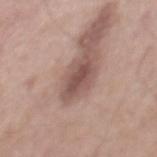<lesion>
<biopsy_status>not biopsied; imaged during a skin examination</biopsy_status>
<lesion_size>
  <long_diameter_mm_approx>4.0</long_diameter_mm_approx>
</lesion_size>
<patient>
  <sex>male</sex>
  <age_approx>55</age_approx>
</patient>
<site>mid back</site>
<image>
  <source>total-body photography crop</source>
  <field_of_view_mm>15</field_of_view_mm>
</image>
<lighting>white-light</lighting>
</lesion>